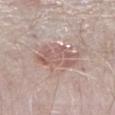anatomic site = the leg
lesion size = ~5 mm (longest diameter)
subject = male, aged around 40
acquisition = ~15 mm crop, total-body skin-cancer survey